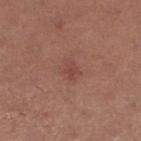notes: no biopsy performed (imaged during a skin exam) | body site: the left lower leg | acquisition: ~15 mm crop, total-body skin-cancer survey | subject: female, aged 43 to 47.